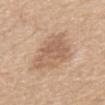Part of a total-body skin-imaging series; this lesion was reviewed on a skin check and was not flagged for biopsy. Measured at roughly 6 mm in maximum diameter. The lesion is located on the front of the torso. The patient is a male approximately 70 years of age. This image is a 15 mm lesion crop taken from a total-body photograph.